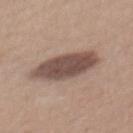biopsy status: imaged on a skin check; not biopsied | subject: female, approximately 55 years of age | illumination: white-light illumination | image: ~15 mm crop, total-body skin-cancer survey | location: the mid back | TBP lesion metrics: a lesion area of about 19 mm² and two-axis asymmetry of about 0.1; a mean CIELAB color near L≈49 a*≈16 b*≈22, a lesion–skin lightness drop of about 14, and a lesion-to-skin contrast of about 10.5 (normalized; higher = more distinct) | diameter: ~7 mm (longest diameter).